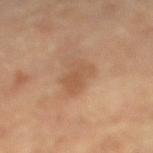The lesion was photographed on a routine skin check and not biopsied; there is no pathology result.